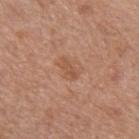Assessment: This lesion was catalogued during total-body skin photography and was not selected for biopsy.